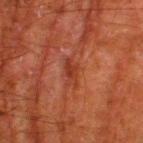Q: Lesion size?
A: ≈3 mm
Q: What kind of image is this?
A: total-body-photography crop, ~15 mm field of view
Q: What are the patient's age and sex?
A: male, aged 78–82
Q: What did automated image analysis measure?
A: a detector confidence of about 90 out of 100 that the crop contains a lesion
Q: What lighting was used for the tile?
A: cross-polarized illumination
Q: What is the anatomic site?
A: the right thigh